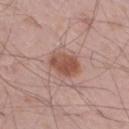Part of a total-body skin-imaging series; this lesion was reviewed on a skin check and was not flagged for biopsy. The lesion is on the right thigh. This is a white-light tile. Cropped from a whole-body photographic skin survey; the tile spans about 15 mm. A male patient, about 55 years old.